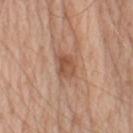| feature | finding |
|---|---|
| automated metrics | a footprint of about 5.5 mm², a shape eccentricity near 0.65, and two-axis asymmetry of about 0.2; a lesion color around L≈52 a*≈22 b*≈31 in CIELAB and about 10 CIELAB-L* units darker than the surrounding skin; lesion-presence confidence of about 100/100 |
| location | the left upper arm |
| image source | ~15 mm tile from a whole-body skin photo |
| lighting | white-light illumination |
| patient | male, about 60 years old |